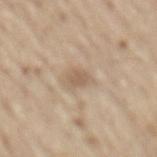The lesion was photographed on a routine skin check and not biopsied; there is no pathology result.
Cropped from a whole-body photographic skin survey; the tile spans about 15 mm.
The lesion is located on the mid back.
A male patient, aged approximately 70.
The recorded lesion diameter is about 2.5 mm.